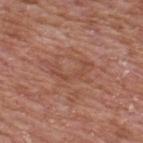Imaged during a routine full-body skin examination; the lesion was not biopsied and no histopathology is available. A male subject roughly 65 years of age. The lesion is on the upper back. The lesion's longest dimension is about 4.5 mm. This is a white-light tile. Cropped from a whole-body photographic skin survey; the tile spans about 15 mm.